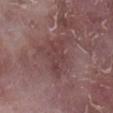{"biopsy_status": "not biopsied; imaged during a skin examination", "patient": {"sex": "male", "age_approx": 75}, "image": {"source": "total-body photography crop", "field_of_view_mm": 15}, "site": "right lower leg"}